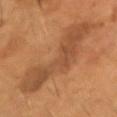  biopsy_status: not biopsied; imaged during a skin examination
  site: head or neck
  image:
    source: total-body photography crop
    field_of_view_mm: 15
  patient:
    sex: male
    age_approx: 55